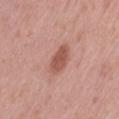Q: Was this lesion biopsied?
A: total-body-photography surveillance lesion; no biopsy
Q: Who is the patient?
A: female, about 55 years old
Q: Lesion location?
A: the left thigh
Q: What kind of image is this?
A: 15 mm crop, total-body photography
Q: What is the lesion's diameter?
A: ~3.5 mm (longest diameter)
Q: What did automated image analysis measure?
A: a lesion area of about 6 mm², an eccentricity of roughly 0.8, and a symmetry-axis asymmetry near 0.2; a border-irregularity rating of about 2/10 and a peripheral color-asymmetry measure near 1